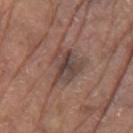Q: Was a biopsy performed?
A: imaged on a skin check; not biopsied
Q: What are the patient's age and sex?
A: male, aged approximately 80
Q: What is the imaging modality?
A: total-body-photography crop, ~15 mm field of view
Q: Lesion size?
A: ≈5 mm
Q: Automated lesion metrics?
A: a border-irregularity index near 5/10, a color-variation rating of about 6.5/10, and peripheral color asymmetry of about 2
Q: Illumination type?
A: white-light illumination
Q: Where on the body is the lesion?
A: the left upper arm